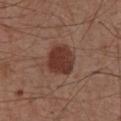No biopsy was performed on this lesion — it was imaged during a full skin examination and was not determined to be concerning. Measured at roughly 4 mm in maximum diameter. The lesion-visualizer software estimated a lesion area of about 11 mm² and a symmetry-axis asymmetry near 0.2. The analysis additionally found an average lesion color of about L≈36 a*≈22 b*≈25 (CIELAB), a lesion–skin lightness drop of about 12, and a normalized lesion–skin contrast near 10. The software also gave an automated nevus-likeness rating near 95 out of 100 and a lesion-detection confidence of about 100/100. The tile uses white-light illumination. A region of skin cropped from a whole-body photographic capture, roughly 15 mm wide. A male subject roughly 55 years of age. Located on the front of the torso.